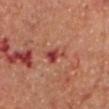Assessment: The lesion was photographed on a routine skin check and not biopsied; there is no pathology result. Acquisition and patient details: Longest diameter approximately 4 mm. An algorithmic analysis of the crop reported a footprint of about 6.5 mm², a shape eccentricity near 0.85, and a symmetry-axis asymmetry near 0.4. The software also gave internal color variation of about 9 on a 0–10 scale and peripheral color asymmetry of about 3. The subject is a male aged 68–72. A lesion tile, about 15 mm wide, cut from a 3D total-body photograph. Imaged with cross-polarized lighting. The lesion is located on the head or neck.